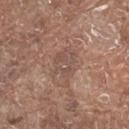Findings:
* notes · no biopsy performed (imaged during a skin exam)
* illumination · white-light illumination
* lesion diameter · ~4.5 mm (longest diameter)
* TBP lesion metrics · a footprint of about 8.5 mm² and two-axis asymmetry of about 0.4; an average lesion color of about L≈50 a*≈18 b*≈24 (CIELAB) and a normalized border contrast of about 5; a color-variation rating of about 3/10 and peripheral color asymmetry of about 1; a nevus-likeness score of about 0/100 and lesion-presence confidence of about 70/100
* subject · male, in their 80s
* site · the back
* imaging modality · ~15 mm crop, total-body skin-cancer survey